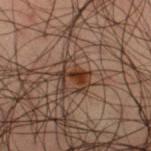patient — male, aged approximately 50 | image — total-body-photography crop, ~15 mm field of view | anatomic site — the right thigh | lesion size — ≈4 mm | TBP lesion metrics — a lesion area of about 6 mm², a shape eccentricity near 0.85, and a shape-asymmetry score of about 0.35 (0 = symmetric); a nevus-likeness score of about 40/100 and lesion-presence confidence of about 100/100 | illumination — cross-polarized.